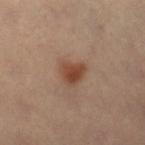<tbp_lesion>
  <biopsy_status>not biopsied; imaged during a skin examination</biopsy_status>
  <image>
    <source>total-body photography crop</source>
    <field_of_view_mm>15</field_of_view_mm>
  </image>
  <patient>
    <sex>female</sex>
    <age_approx>40</age_approx>
  </patient>
  <lighting>cross-polarized</lighting>
  <site>left leg</site>
  <lesion_size>
    <long_diameter_mm_approx>3.0</long_diameter_mm_approx>
  </lesion_size>
</tbp_lesion>